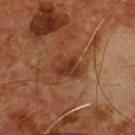Located on the chest.
Longest diameter approximately 3.5 mm.
The subject is a male approximately 55 years of age.
A 15 mm crop from a total-body photograph taken for skin-cancer surveillance.
Imaged with cross-polarized lighting.